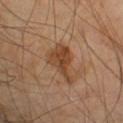Impression: This lesion was catalogued during total-body skin photography and was not selected for biopsy. Background: The lesion's longest dimension is about 5 mm. Automated tile analysis of the lesion measured a lesion area of about 9 mm² and a shape eccentricity near 0.85. The analysis additionally found a mean CIELAB color near L≈44 a*≈21 b*≈33 and about 10 CIELAB-L* units darker than the surrounding skin. It also reported border irregularity of about 6 on a 0–10 scale, a color-variation rating of about 4/10, and radial color variation of about 1.5. The analysis additionally found a classifier nevus-likeness of about 60/100 and a lesion-detection confidence of about 100/100. The patient is a male aged around 65. On the left upper arm. A roughly 15 mm field-of-view crop from a total-body skin photograph.